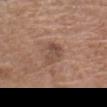follow-up: no biopsy performed (imaged during a skin exam) | subject: male, aged 63 to 67 | image source: total-body-photography crop, ~15 mm field of view | site: the head or neck.